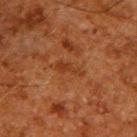Q: Is there a histopathology result?
A: total-body-photography surveillance lesion; no biopsy
Q: What are the patient's age and sex?
A: male, aged approximately 60
Q: Illumination type?
A: cross-polarized
Q: How was this image acquired?
A: 15 mm crop, total-body photography
Q: Lesion size?
A: ~3.5 mm (longest diameter)
Q: What is the anatomic site?
A: the upper back